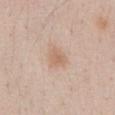Recorded during total-body skin imaging; not selected for excision or biopsy. Captured under white-light illumination. Automated tile analysis of the lesion measured a border-irregularity rating of about 1.5/10, a within-lesion color-variation index near 2/10, and peripheral color asymmetry of about 0.5. A 15 mm close-up tile from a total-body photography series done for melanoma screening. The patient is a male roughly 55 years of age. The lesion is located on the abdomen.